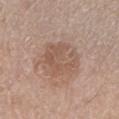No biopsy was performed on this lesion — it was imaged during a full skin examination and was not determined to be concerning.